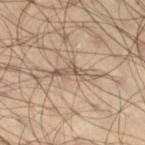Imaged during a routine full-body skin examination; the lesion was not biopsied and no histopathology is available. The recorded lesion diameter is about 5 mm. The lesion is located on the right thigh. Automated tile analysis of the lesion measured a shape eccentricity near 0.95 and two-axis asymmetry of about 0.7. And it measured a lesion color around L≈54 a*≈13 b*≈27 in CIELAB and roughly 9 lightness units darker than nearby skin. A 15 mm crop from a total-body photograph taken for skin-cancer surveillance. A male subject aged around 45.